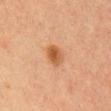| field | value |
|---|---|
| follow-up | total-body-photography surveillance lesion; no biopsy |
| image | 15 mm crop, total-body photography |
| subject | male, aged 33 to 37 |
| anatomic site | the front of the torso |
| illumination | cross-polarized |
| automated lesion analysis | a lesion color around L≈50 a*≈23 b*≈37 in CIELAB and a lesion-to-skin contrast of about 8.5 (normalized; higher = more distinct); a classifier nevus-likeness of about 95/100 |
| lesion diameter | about 3 mm |